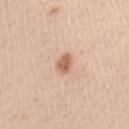follow-up = catalogued during a skin exam; not biopsied